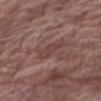| key | value |
|---|---|
| notes | total-body-photography surveillance lesion; no biopsy |
| subject | male, roughly 80 years of age |
| anatomic site | the right thigh |
| acquisition | ~15 mm crop, total-body skin-cancer survey |